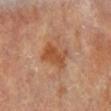Q: Was a biopsy performed?
A: total-body-photography surveillance lesion; no biopsy
Q: What kind of image is this?
A: ~15 mm crop, total-body skin-cancer survey
Q: What is the anatomic site?
A: the left lower leg
Q: Who is the patient?
A: female, aged 73 to 77